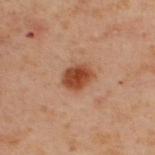This lesion was catalogued during total-body skin photography and was not selected for biopsy. On the upper back. Automated tile analysis of the lesion measured an eccentricity of roughly 0.6 and a symmetry-axis asymmetry near 0.15. The analysis additionally found a border-irregularity rating of about 1.5/10 and a within-lesion color-variation index near 3/10. A male subject, aged around 50. Cropped from a whole-body photographic skin survey; the tile spans about 15 mm.